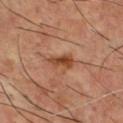{
  "biopsy_status": "not biopsied; imaged during a skin examination",
  "patient": {
    "sex": "male",
    "age_approx": 55
  },
  "image": {
    "source": "total-body photography crop",
    "field_of_view_mm": 15
  },
  "lesion_size": {
    "long_diameter_mm_approx": 3.5
  },
  "lighting": "cross-polarized",
  "site": "chest"
}